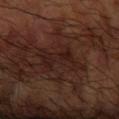Clinical impression: Recorded during total-body skin imaging; not selected for excision or biopsy.